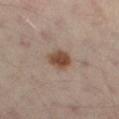No biopsy was performed on this lesion — it was imaged during a full skin examination and was not determined to be concerning. The total-body-photography lesion software estimated an automated nevus-likeness rating near 100 out of 100 and a detector confidence of about 100 out of 100 that the crop contains a lesion. The lesion is on the left thigh. A lesion tile, about 15 mm wide, cut from a 3D total-body photograph. The recorded lesion diameter is about 3 mm. A subject aged approximately 55.